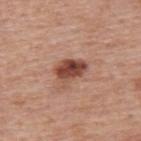The lesion was photographed on a routine skin check and not biopsied; there is no pathology result.
A 15 mm close-up tile from a total-body photography series done for melanoma screening.
Captured under white-light illumination.
The lesion-visualizer software estimated an area of roughly 8 mm² and two-axis asymmetry of about 0.3. The analysis additionally found a lesion color around L≈47 a*≈24 b*≈28 in CIELAB and a normalized lesion–skin contrast near 10.5.
A male subject, about 75 years old.
The lesion's longest dimension is about 4 mm.
The lesion is on the upper back.